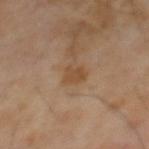{
  "biopsy_status": "not biopsied; imaged during a skin examination",
  "lighting": "cross-polarized",
  "patient": {
    "sex": "male",
    "age_approx": 65
  },
  "image": {
    "source": "total-body photography crop",
    "field_of_view_mm": 15
  },
  "automated_metrics": {
    "eccentricity": 0.7,
    "shape_asymmetry": 0.3,
    "vs_skin_darker_L": 7.0,
    "vs_skin_contrast_norm": 7.0
  }
}